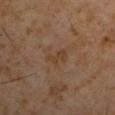Q: Was this lesion biopsied?
A: total-body-photography surveillance lesion; no biopsy
Q: What is the lesion's diameter?
A: ~3 mm (longest diameter)
Q: Who is the patient?
A: male, in their 60s
Q: What is the imaging modality?
A: 15 mm crop, total-body photography
Q: Where on the body is the lesion?
A: the left upper arm
Q: How was the tile lit?
A: cross-polarized
Q: What did automated image analysis measure?
A: a lesion area of about 3 mm², an eccentricity of roughly 0.9, and a shape-asymmetry score of about 0.4 (0 = symmetric)